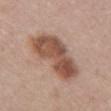Captured during whole-body skin photography for melanoma surveillance; the lesion was not biopsied. Automated tile analysis of the lesion measured a lesion area of about 22 mm², an outline eccentricity of about 0.9 (0 = round, 1 = elongated), and two-axis asymmetry of about 0.25. And it measured a mean CIELAB color near L≈51 a*≈20 b*≈28. And it measured a nevus-likeness score of about 5/100 and lesion-presence confidence of about 100/100. A female patient, aged around 50. The lesion is on the left upper arm. Cropped from a whole-body photographic skin survey; the tile spans about 15 mm.